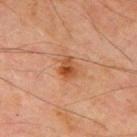Clinical impression: The lesion was photographed on a routine skin check and not biopsied; there is no pathology result. Image and clinical context: A male patient, about 70 years old. On the chest. Longest diameter approximately 2.5 mm. Cropped from a total-body skin-imaging series; the visible field is about 15 mm.